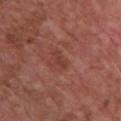<tbp_lesion>
  <biopsy_status>not biopsied; imaged during a skin examination</biopsy_status>
  <automated_metrics>
    <area_mm2_approx>2.0</area_mm2_approx>
    <eccentricity>0.95</eccentricity>
    <shape_asymmetry>0.35</shape_asymmetry>
    <nevus_likeness_0_100>0</nevus_likeness_0_100>
  </automated_metrics>
  <patient>
    <sex>male</sex>
    <age_approx>75</age_approx>
  </patient>
  <site>chest</site>
  <image>
    <source>total-body photography crop</source>
    <field_of_view_mm>15</field_of_view_mm>
  </image>
  <lighting>white-light</lighting>
</tbp_lesion>